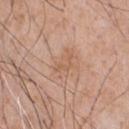Q: What are the patient's age and sex?
A: male, in their mid- to late 50s
Q: How was the tile lit?
A: white-light illumination
Q: Lesion location?
A: the chest
Q: How large is the lesion?
A: about 3 mm
Q: What did automated image analysis measure?
A: a lesion-to-skin contrast of about 5 (normalized; higher = more distinct); a border-irregularity index near 8/10, a color-variation rating of about 0/10, and peripheral color asymmetry of about 0
Q: How was this image acquired?
A: ~15 mm tile from a whole-body skin photo